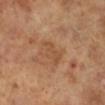notes = no biopsy performed (imaged during a skin exam); image source = total-body-photography crop, ~15 mm field of view; body site = the leg; subject = female, roughly 70 years of age; lesion size = about 4 mm.